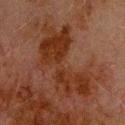Assessment:
The lesion was photographed on a routine skin check and not biopsied; there is no pathology result.
Context:
This is a cross-polarized tile. About 10.5 mm across. A male subject, aged approximately 80. A region of skin cropped from a whole-body photographic capture, roughly 15 mm wide. Located on the upper back.